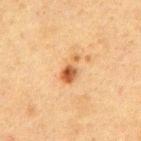notes: catalogued during a skin exam; not biopsied
site: the front of the torso
patient: male, aged approximately 75
image: 15 mm crop, total-body photography
diameter: ~3.5 mm (longest diameter)
illumination: cross-polarized